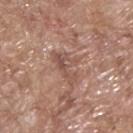Imaged during a routine full-body skin examination; the lesion was not biopsied and no histopathology is available.
A male subject about 70 years old.
The lesion is on the upper back.
A region of skin cropped from a whole-body photographic capture, roughly 15 mm wide.
Imaged with white-light lighting.
The lesion-visualizer software estimated an outline eccentricity of about 0.85 (0 = round, 1 = elongated) and a symmetry-axis asymmetry near 0.45. And it measured an average lesion color of about L≈52 a*≈20 b*≈25 (CIELAB), a lesion–skin lightness drop of about 8, and a lesion-to-skin contrast of about 6 (normalized; higher = more distinct). The software also gave border irregularity of about 6.5 on a 0–10 scale, internal color variation of about 5.5 on a 0–10 scale, and a peripheral color-asymmetry measure near 2.